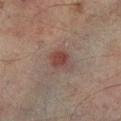Acquisition and patient details: Measured at roughly 3 mm in maximum diameter. Captured under cross-polarized illumination. A close-up tile cropped from a whole-body skin photograph, about 15 mm across. An algorithmic analysis of the crop reported a lesion color around L≈36 a*≈17 b*≈19 in CIELAB, a lesion–skin lightness drop of about 7, and a normalized border contrast of about 7. It also reported border irregularity of about 3 on a 0–10 scale, a color-variation rating of about 3.5/10, and peripheral color asymmetry of about 1. It also reported a nevus-likeness score of about 85/100 and a lesion-detection confidence of about 100/100. From the left lower leg. A male patient, aged approximately 75.